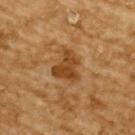<record>
  <biopsy_status>not biopsied; imaged during a skin examination</biopsy_status>
  <lighting>cross-polarized</lighting>
  <image>
    <source>total-body photography crop</source>
    <field_of_view_mm>15</field_of_view_mm>
  </image>
  <patient>
    <sex>male</sex>
    <age_approx>85+</age_approx>
  </patient>
  <site>upper back</site>
  <automated_metrics>
    <eccentricity>0.6</eccentricity>
    <shape_asymmetry>0.5</shape_asymmetry>
  </automated_metrics>
</record>